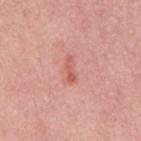A male patient in their 60s.
The recorded lesion diameter is about 3 mm.
Captured under white-light illumination.
On the mid back.
A 15 mm close-up tile from a total-body photography series done for melanoma screening.